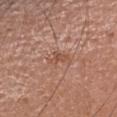Case summary:
– workup · imaged on a skin check; not biopsied
– acquisition · 15 mm crop, total-body photography
– patient · female, aged around 25
– lesion size · about 3 mm
– lighting · white-light
– body site · the head or neck